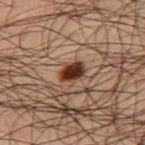notes: total-body-photography surveillance lesion; no biopsy
patient: male, in their 50s
acquisition: total-body-photography crop, ~15 mm field of view
lighting: cross-polarized illumination
lesion size: about 3 mm
automated metrics: an area of roughly 5 mm² and an eccentricity of roughly 0.7; a mean CIELAB color near L≈25 a*≈17 b*≈22 and about 14 CIELAB-L* units darker than the surrounding skin; radial color variation of about 1; a nevus-likeness score of about 100/100 and lesion-presence confidence of about 100/100
body site: the right thigh